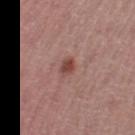Captured during whole-body skin photography for melanoma surveillance; the lesion was not biopsied. Located on the left thigh. A female subject aged around 55. A region of skin cropped from a whole-body photographic capture, roughly 15 mm wide. Imaged with white-light lighting. The recorded lesion diameter is about 2.5 mm.